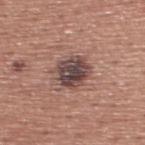Recorded during total-body skin imaging; not selected for excision or biopsy. Imaged with white-light lighting. From the upper back. A 15 mm close-up extracted from a 3D total-body photography capture. The subject is a male in their mid-40s.